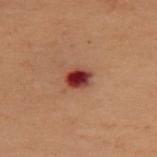- biopsy status: imaged on a skin check; not biopsied
- site: the upper back
- image source: ~15 mm crop, total-body skin-cancer survey
- TBP lesion metrics: an average lesion color of about L≈34 a*≈26 b*≈25 (CIELAB), a lesion–skin lightness drop of about 14, and a normalized lesion–skin contrast near 12.5; border irregularity of about 1.5 on a 0–10 scale, internal color variation of about 7 on a 0–10 scale, and a peripheral color-asymmetry measure near 2
- size: ~3 mm (longest diameter)
- subject: female, aged 48 to 52